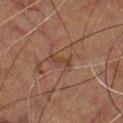Clinical impression:
Imaged during a routine full-body skin examination; the lesion was not biopsied and no histopathology is available.
Image and clinical context:
A male patient, aged around 50. The lesion is located on the front of the torso. Imaged with cross-polarized lighting. Measured at roughly 3 mm in maximum diameter. An algorithmic analysis of the crop reported a classifier nevus-likeness of about 0/100 and lesion-presence confidence of about 80/100. A region of skin cropped from a whole-body photographic capture, roughly 15 mm wide.